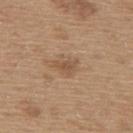This lesion was catalogued during total-body skin photography and was not selected for biopsy. Located on the upper back. Measured at roughly 3 mm in maximum diameter. Captured under white-light illumination. A male patient, approximately 65 years of age. A roughly 15 mm field-of-view crop from a total-body skin photograph.